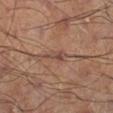Impression: No biopsy was performed on this lesion — it was imaged during a full skin examination and was not determined to be concerning. Clinical summary: The lesion is on the left thigh. A roughly 15 mm field-of-view crop from a total-body skin photograph.